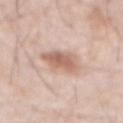follow-up: total-body-photography surveillance lesion; no biopsy
imaging modality: 15 mm crop, total-body photography
location: the abdomen
subject: male, about 80 years old